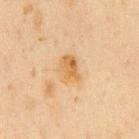The lesion was photographed on a routine skin check and not biopsied; there is no pathology result. The patient is a male in their 60s. From the chest. Longest diameter approximately 3.5 mm. This is a cross-polarized tile. A 15 mm crop from a total-body photograph taken for skin-cancer surveillance.